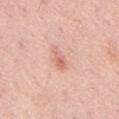notes = catalogued during a skin exam; not biopsied
subject = male, aged 63–67
lesion size = ≈3 mm
image source = 15 mm crop, total-body photography
site = the mid back
tile lighting = white-light